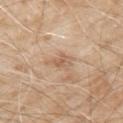Q: Was a biopsy performed?
A: imaged on a skin check; not biopsied
Q: How large is the lesion?
A: about 3 mm
Q: Illumination type?
A: white-light
Q: What are the patient's age and sex?
A: male, aged approximately 80
Q: What is the anatomic site?
A: the upper back
Q: What kind of image is this?
A: 15 mm crop, total-body photography